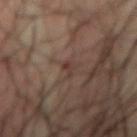Assessment:
This lesion was catalogued during total-body skin photography and was not selected for biopsy.
Clinical summary:
A region of skin cropped from a whole-body photographic capture, roughly 15 mm wide. Located on the front of the torso. Approximately 2.5 mm at its widest. A male subject, aged approximately 70.